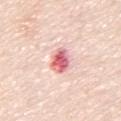<record>
  <image>
    <source>total-body photography crop</source>
    <field_of_view_mm>15</field_of_view_mm>
  </image>
  <automated_metrics>
    <cielab_L>67</cielab_L>
    <cielab_a>33</cielab_a>
    <cielab_b>22</cielab_b>
    <vs_skin_darker_L>17.0</vs_skin_darker_L>
    <vs_skin_contrast_norm>9.5</vs_skin_contrast_norm>
    <border_irregularity_0_10>2.0</border_irregularity_0_10>
    <peripheral_color_asymmetry>2.5</peripheral_color_asymmetry>
  </automated_metrics>
  <lesion_size>
    <long_diameter_mm_approx>3.5</long_diameter_mm_approx>
  </lesion_size>
  <site>front of the torso</site>
  <lighting>white-light</lighting>
  <patient>
    <sex>male</sex>
    <age_approx>80</age_approx>
  </patient>
</record>